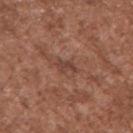This lesion was catalogued during total-body skin photography and was not selected for biopsy. A 15 mm close-up extracted from a 3D total-body photography capture. The patient is a male in their mid- to late 40s. On the upper back.